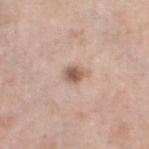Part of a total-body skin-imaging series; this lesion was reviewed on a skin check and was not flagged for biopsy.
On the left thigh.
Approximately 2.5 mm at its widest.
Imaged with white-light lighting.
A female subject aged approximately 55.
A 15 mm close-up extracted from a 3D total-body photography capture.